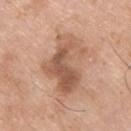| key | value |
|---|---|
| follow-up | imaged on a skin check; not biopsied |
| acquisition | 15 mm crop, total-body photography |
| subject | male, about 75 years old |
| TBP lesion metrics | a classifier nevus-likeness of about 0/100 and a lesion-detection confidence of about 100/100 |
| location | the upper back |